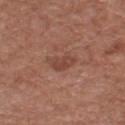{"biopsy_status": "not biopsied; imaged during a skin examination", "patient": {"sex": "male", "age_approx": 75}, "site": "chest", "image": {"source": "total-body photography crop", "field_of_view_mm": 15}}